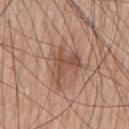Q: Is there a histopathology result?
A: imaged on a skin check; not biopsied
Q: How large is the lesion?
A: ≈5 mm
Q: What kind of image is this?
A: ~15 mm crop, total-body skin-cancer survey
Q: Who is the patient?
A: male, aged around 80
Q: Automated lesion metrics?
A: a lesion color around L≈51 a*≈21 b*≈28 in CIELAB and about 10 CIELAB-L* units darker than the surrounding skin; an automated nevus-likeness rating near 10 out of 100
Q: Illumination type?
A: white-light illumination
Q: What is the anatomic site?
A: the chest